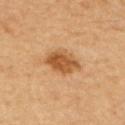Findings:
* notes · catalogued during a skin exam; not biopsied
* site · the upper back
* patient · male, aged approximately 65
* diameter · about 4.5 mm
* image · ~15 mm tile from a whole-body skin photo